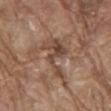This lesion was catalogued during total-body skin photography and was not selected for biopsy. This is a white-light tile. Automated image analysis of the tile measured an automated nevus-likeness rating near 0 out of 100 and lesion-presence confidence of about 60/100. Located on the abdomen. A region of skin cropped from a whole-body photographic capture, roughly 15 mm wide. About 6 mm across. A male subject, aged 78–82.